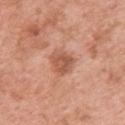Notes:
• biopsy status · total-body-photography surveillance lesion; no biopsy
• patient · female, about 50 years old
• site · the upper back
• acquisition · ~15 mm crop, total-body skin-cancer survey
• image-analysis metrics · a lesion color around L≈56 a*≈25 b*≈32 in CIELAB, a lesion–skin lightness drop of about 11, and a normalized border contrast of about 7; border irregularity of about 2 on a 0–10 scale, a color-variation rating of about 3.5/10, and a peripheral color-asymmetry measure near 1; a classifier nevus-likeness of about 5/100 and a detector confidence of about 100 out of 100 that the crop contains a lesion
• lesion size · about 3 mm
• tile lighting · white-light illumination